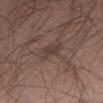Background:
A roughly 15 mm field-of-view crop from a total-body skin photograph. Automated image analysis of the tile measured a lesion area of about 4 mm², an eccentricity of roughly 0.75, and two-axis asymmetry of about 0.4. It also reported a border-irregularity rating of about 4.5/10, a within-lesion color-variation index near 2/10, and peripheral color asymmetry of about 0.5. From the abdomen. The patient is a male roughly 75 years of age.